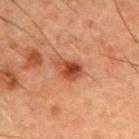Impression:
The lesion was tiled from a total-body skin photograph and was not biopsied.
Image and clinical context:
Cropped from a total-body skin-imaging series; the visible field is about 15 mm. A male subject, about 50 years old. From the upper back.